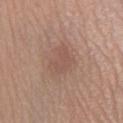Part of a total-body skin-imaging series; this lesion was reviewed on a skin check and was not flagged for biopsy.
A 15 mm crop from a total-body photograph taken for skin-cancer surveillance.
A female patient aged 43–47.
The lesion is on the right lower leg.